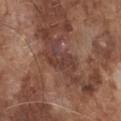Q: What is the anatomic site?
A: the front of the torso
Q: How was this image acquired?
A: ~15 mm crop, total-body skin-cancer survey
Q: Who is the patient?
A: male, in their mid- to late 70s